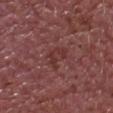biopsy status: catalogued during a skin exam; not biopsied
anatomic site: the front of the torso
image-analysis metrics: two-axis asymmetry of about 0.65; about 6 CIELAB-L* units darker than the surrounding skin; border irregularity of about 9 on a 0–10 scale and radial color variation of about 0
illumination: white-light
acquisition: total-body-photography crop, ~15 mm field of view
lesion diameter: ≈3.5 mm
subject: male, in their mid- to late 60s